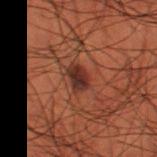Q: What kind of image is this?
A: 15 mm crop, total-body photography
Q: Patient demographics?
A: male, aged approximately 50
Q: What did automated image analysis measure?
A: a border-irregularity index near 1.5/10 and a within-lesion color-variation index near 4.5/10; lesion-presence confidence of about 100/100
Q: Where on the body is the lesion?
A: the left thigh